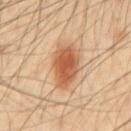{
  "biopsy_status": "not biopsied; imaged during a skin examination",
  "automated_metrics": {
    "area_mm2_approx": 14.0,
    "cielab_L": 58,
    "cielab_a": 24,
    "cielab_b": 36,
    "vs_skin_darker_L": 13.0,
    "vs_skin_contrast_norm": 9.0,
    "border_irregularity_0_10": 1.5,
    "color_variation_0_10": 5.0,
    "peripheral_color_asymmetry": 1.5,
    "nevus_likeness_0_100": 100,
    "lesion_detection_confidence_0_100": 100
  },
  "image": {
    "source": "total-body photography crop",
    "field_of_view_mm": 15
  },
  "patient": {
    "sex": "male",
    "age_approx": 60
  },
  "site": "chest",
  "lesion_size": {
    "long_diameter_mm_approx": 5.5
  },
  "lighting": "cross-polarized"
}